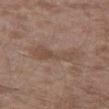The lesion was tiled from a total-body skin photograph and was not biopsied.
The recorded lesion diameter is about 6 mm.
Imaged with white-light lighting.
Automated image analysis of the tile measured border irregularity of about 6 on a 0–10 scale, internal color variation of about 3 on a 0–10 scale, and radial color variation of about 1. The analysis additionally found a nevus-likeness score of about 0/100 and lesion-presence confidence of about 100/100.
A male subject, in their mid- to late 40s.
From the left thigh.
This image is a 15 mm lesion crop taken from a total-body photograph.